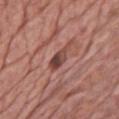The lesion was tiled from a total-body skin photograph and was not biopsied. The lesion is located on the chest. A male patient in their mid- to late 80s or older. Captured under white-light illumination. Cropped from a total-body skin-imaging series; the visible field is about 15 mm.